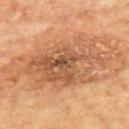Recorded during total-body skin imaging; not selected for excision or biopsy.
This image is a 15 mm lesion crop taken from a total-body photograph.
The lesion is on the mid back.
The lesion-visualizer software estimated a border-irregularity index near 5.5/10, a within-lesion color-variation index near 6.5/10, and peripheral color asymmetry of about 2. The software also gave an automated nevus-likeness rating near 25 out of 100 and lesion-presence confidence of about 100/100.
The tile uses cross-polarized illumination.
A male subject, in their mid-60s.